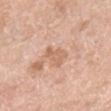Part of a total-body skin-imaging series; this lesion was reviewed on a skin check and was not flagged for biopsy.
The lesion-visualizer software estimated a normalized lesion–skin contrast near 5.5. The software also gave border irregularity of about 4 on a 0–10 scale and internal color variation of about 1.5 on a 0–10 scale. It also reported a classifier nevus-likeness of about 0/100 and a lesion-detection confidence of about 100/100.
On the right lower leg.
A lesion tile, about 15 mm wide, cut from a 3D total-body photograph.
A female patient, aged 68 to 72.